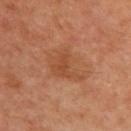Findings:
* workup: imaged on a skin check; not biopsied
* image source: 15 mm crop, total-body photography
* subject: male, about 50 years old
* image-analysis metrics: a lesion color around L≈48 a*≈24 b*≈35 in CIELAB and a lesion–skin lightness drop of about 7; a color-variation rating of about 2.5/10
* diameter: ≈4.5 mm
* location: the back